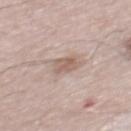Part of a total-body skin-imaging series; this lesion was reviewed on a skin check and was not flagged for biopsy. A male patient aged approximately 55. This image is a 15 mm lesion crop taken from a total-body photograph. Automated image analysis of the tile measured a footprint of about 4.5 mm². And it measured border irregularity of about 2.5 on a 0–10 scale, a color-variation rating of about 2/10, and a peripheral color-asymmetry measure near 1. It also reported a detector confidence of about 90 out of 100 that the crop contains a lesion.